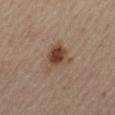{"biopsy_status": "not biopsied; imaged during a skin examination", "automated_metrics": {"cielab_L": 44, "cielab_a": 18, "cielab_b": 27, "vs_skin_darker_L": 11.0, "border_irregularity_0_10": 4.0, "color_variation_0_10": 6.0}, "site": "left thigh", "patient": {"sex": "male", "age_approx": 65}, "image": {"source": "total-body photography crop", "field_of_view_mm": 15}, "lesion_size": {"long_diameter_mm_approx": 3.5}}